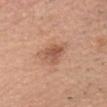Impression:
Imaged during a routine full-body skin examination; the lesion was not biopsied and no histopathology is available.
Acquisition and patient details:
The patient is a male in their 40s. About 3 mm across. On the head or neck. A 15 mm close-up tile from a total-body photography series done for melanoma screening. Imaged with white-light lighting.